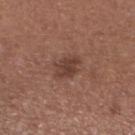Clinical impression: Captured during whole-body skin photography for melanoma surveillance; the lesion was not biopsied. Clinical summary: A close-up tile cropped from a whole-body skin photograph, about 15 mm across. A female patient in their mid- to late 50s. About 3 mm across. This is a white-light tile. From the left lower leg.